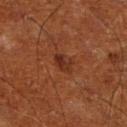No biopsy was performed on this lesion — it was imaged during a full skin examination and was not determined to be concerning.
Captured under cross-polarized illumination.
A male subject roughly 65 years of age.
A 15 mm crop from a total-body photograph taken for skin-cancer surveillance.
On the leg.
About 2.5 mm across.